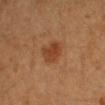Assessment:
Part of a total-body skin-imaging series; this lesion was reviewed on a skin check and was not flagged for biopsy.
Context:
The patient is a female aged approximately 40. About 3.5 mm across. Captured under cross-polarized illumination. A lesion tile, about 15 mm wide, cut from a 3D total-body photograph. On the left upper arm.